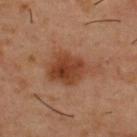Clinical impression:
Recorded during total-body skin imaging; not selected for excision or biopsy.
Background:
A 15 mm close-up extracted from a 3D total-body photography capture. A male subject, about 55 years old. Approximately 6 mm at its widest. Imaged with cross-polarized lighting. Located on the upper back.